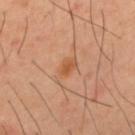notes: no biopsy performed (imaged during a skin exam) | automated lesion analysis: a footprint of about 3.5 mm², an outline eccentricity of about 0.8 (0 = round, 1 = elongated), and two-axis asymmetry of about 0.25; a mean CIELAB color near L≈54 a*≈24 b*≈37, a lesion–skin lightness drop of about 8, and a normalized lesion–skin contrast near 6.5; a border-irregularity index near 2.5/10, internal color variation of about 2 on a 0–10 scale, and peripheral color asymmetry of about 0.5 | anatomic site: the mid back | subject: male, in their 40s | image: total-body-photography crop, ~15 mm field of view.